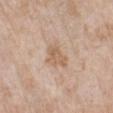notes: imaged on a skin check; not biopsied | tile lighting: white-light | patient: female, aged 68–72 | diameter: ~3 mm (longest diameter) | imaging modality: ~15 mm crop, total-body skin-cancer survey | automated metrics: an area of roughly 5.5 mm² and a shape-asymmetry score of about 0.45 (0 = symmetric); border irregularity of about 4.5 on a 0–10 scale, a color-variation rating of about 2/10, and peripheral color asymmetry of about 0.5; lesion-presence confidence of about 100/100 | site: the left lower leg.